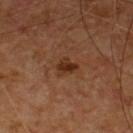Clinical impression: Imaged during a routine full-body skin examination; the lesion was not biopsied and no histopathology is available. Image and clinical context: This is a cross-polarized tile. The patient is a male in their mid- to late 50s. A 15 mm crop from a total-body photograph taken for skin-cancer surveillance. On the front of the torso. Automated tile analysis of the lesion measured a lesion color around L≈27 a*≈20 b*≈28 in CIELAB, a lesion–skin lightness drop of about 9, and a normalized border contrast of about 9. And it measured a classifier nevus-likeness of about 80/100 and a lesion-detection confidence of about 100/100.